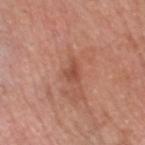Impression: No biopsy was performed on this lesion — it was imaged during a full skin examination and was not determined to be concerning. Acquisition and patient details: A male patient about 80 years old. On the head or neck. Approximately 2.5 mm at its widest. Automated tile analysis of the lesion measured border irregularity of about 4.5 on a 0–10 scale and a peripheral color-asymmetry measure near 0.5. It also reported a classifier nevus-likeness of about 0/100 and a detector confidence of about 100 out of 100 that the crop contains a lesion. This is a white-light tile. Cropped from a whole-body photographic skin survey; the tile spans about 15 mm.